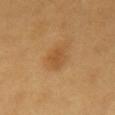Q: Is there a histopathology result?
A: total-body-photography surveillance lesion; no biopsy
Q: Where on the body is the lesion?
A: the chest
Q: What is the lesion's diameter?
A: about 3.5 mm
Q: What did automated image analysis measure?
A: a border-irregularity rating of about 3/10 and internal color variation of about 2.5 on a 0–10 scale; a classifier nevus-likeness of about 80/100
Q: What are the patient's age and sex?
A: male, aged 58 to 62
Q: How was this image acquired?
A: ~15 mm tile from a whole-body skin photo
Q: Illumination type?
A: cross-polarized illumination